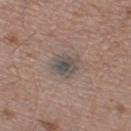Notes:
* biopsy status · total-body-photography surveillance lesion; no biopsy
* image-analysis metrics · an area of roughly 6.5 mm², an outline eccentricity of about 0.4 (0 = round, 1 = elongated), and a symmetry-axis asymmetry near 0.2; a mean CIELAB color near L≈48 a*≈8 b*≈16, roughly 9 lightness units darker than nearby skin, and a normalized lesion–skin contrast near 8.5; border irregularity of about 2.5 on a 0–10 scale, a color-variation rating of about 5.5/10, and radial color variation of about 2; a classifier nevus-likeness of about 0/100 and lesion-presence confidence of about 95/100
* lighting · white-light illumination
* size · ~3 mm (longest diameter)
* image · 15 mm crop, total-body photography
* subject · male, in their mid-40s
* anatomic site · the leg